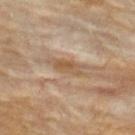biopsy_status: not biopsied; imaged during a skin examination
patient:
  sex: female
  age_approx: 60
image:
  source: total-body photography crop
  field_of_view_mm: 15
site: upper back
lesion_size:
  long_diameter_mm_approx: 3.5